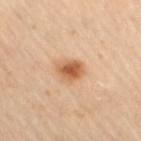Image and clinical context:
A male subject aged around 65. From the right thigh. Cropped from a total-body skin-imaging series; the visible field is about 15 mm.